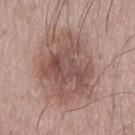biopsy status: imaged on a skin check; not biopsied
location: the left thigh
lesion size: ≈8.5 mm
TBP lesion metrics: a footprint of about 36 mm², a shape eccentricity near 0.65, and a shape-asymmetry score of about 0.2 (0 = symmetric); a lesion color around L≈52 a*≈18 b*≈23 in CIELAB and a normalized border contrast of about 7.5
acquisition: total-body-photography crop, ~15 mm field of view
patient: male, about 65 years old
tile lighting: white-light illumination